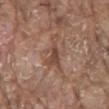Clinical impression:
The lesion was photographed on a routine skin check and not biopsied; there is no pathology result.
Clinical summary:
From the chest. The lesion-visualizer software estimated a classifier nevus-likeness of about 0/100. Cropped from a total-body skin-imaging series; the visible field is about 15 mm. The lesion's longest dimension is about 5 mm. A male patient in their 80s. This is a white-light tile.